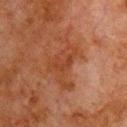The lesion was tiled from a total-body skin photograph and was not biopsied.
The lesion-visualizer software estimated a lesion area of about 14 mm², an eccentricity of roughly 0.85, and a symmetry-axis asymmetry near 0.6. And it measured a border-irregularity index near 9.5/10 and a within-lesion color-variation index near 2.5/10.
The recorded lesion diameter is about 6.5 mm.
A male patient about 80 years old.
From the front of the torso.
A 15 mm close-up tile from a total-body photography series done for melanoma screening.